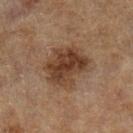Case summary:
- follow-up — imaged on a skin check; not biopsied
- subject — male, aged 83 or over
- tile lighting — cross-polarized illumination
- size — about 5 mm
- body site — the left lower leg
- image — ~15 mm tile from a whole-body skin photo
- automated lesion analysis — about 12 CIELAB-L* units darker than the surrounding skin